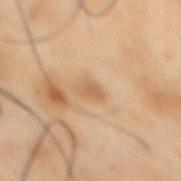Part of a total-body skin-imaging series; this lesion was reviewed on a skin check and was not flagged for biopsy. This image is a 15 mm lesion crop taken from a total-body photograph. Located on the front of the torso. Automated image analysis of the tile measured a normalized border contrast of about 5.5. It also reported a classifier nevus-likeness of about 5/100 and lesion-presence confidence of about 100/100. The patient is a male aged 48–52. Measured at roughly 2.5 mm in maximum diameter. The tile uses cross-polarized illumination.